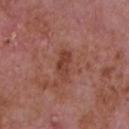{"biopsy_status": "not biopsied; imaged during a skin examination", "lighting": "white-light", "patient": {"sex": "male", "age_approx": 65}, "site": "chest", "lesion_size": {"long_diameter_mm_approx": 4.0}, "image": {"source": "total-body photography crop", "field_of_view_mm": 15}, "automated_metrics": {"border_irregularity_0_10": 4.0, "color_variation_0_10": 2.5, "peripheral_color_asymmetry": 1.0, "nevus_likeness_0_100": 0, "lesion_detection_confidence_0_100": 100}}